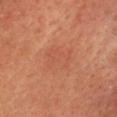This is a cross-polarized tile. This image is a 15 mm lesion crop taken from a total-body photograph. The patient is roughly 70 years of age. The lesion is located on the head or neck. Measured at roughly 4 mm in maximum diameter.